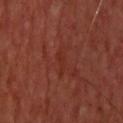| feature | finding |
|---|---|
| notes | catalogued during a skin exam; not biopsied |
| imaging modality | 15 mm crop, total-body photography |
| body site | the head or neck |
| patient | male, approximately 65 years of age |